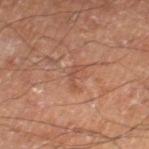Imaged during a routine full-body skin examination; the lesion was not biopsied and no histopathology is available. The recorded lesion diameter is about 2.5 mm. A male patient in their 60s. An algorithmic analysis of the crop reported an outline eccentricity of about 0.9 (0 = round, 1 = elongated) and a shape-asymmetry score of about 0.55 (0 = symmetric). The analysis additionally found a mean CIELAB color near L≈45 a*≈21 b*≈28, a lesion–skin lightness drop of about 6, and a normalized border contrast of about 5. And it measured a lesion-detection confidence of about 100/100. A 15 mm crop from a total-body photograph taken for skin-cancer surveillance. Located on the right thigh. Imaged with cross-polarized lighting.